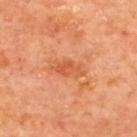| key | value |
|---|---|
| biopsy status | catalogued during a skin exam; not biopsied |
| patient | male, aged around 65 |
| body site | the upper back |
| image-analysis metrics | an average lesion color of about L≈57 a*≈32 b*≈41 (CIELAB) and a normalized lesion–skin contrast near 5.5 |
| image | total-body-photography crop, ~15 mm field of view |
| lighting | cross-polarized |